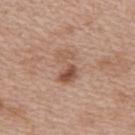Impression: Part of a total-body skin-imaging series; this lesion was reviewed on a skin check and was not flagged for biopsy. Image and clinical context: Measured at roughly 4 mm in maximum diameter. A female patient, about 40 years old. The lesion is located on the right upper arm. The lesion-visualizer software estimated a border-irregularity index near 5/10, internal color variation of about 5.5 on a 0–10 scale, and radial color variation of about 2. And it measured a classifier nevus-likeness of about 45/100. This is a white-light tile. A close-up tile cropped from a whole-body skin photograph, about 15 mm across.